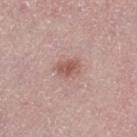The lesion was tiled from a total-body skin photograph and was not biopsied.
On the leg.
Cropped from a whole-body photographic skin survey; the tile spans about 15 mm.
A female patient roughly 65 years of age.
Imaged with white-light lighting.